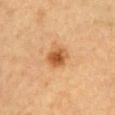Findings:
• follow-up: imaged on a skin check; not biopsied
• patient: female, about 30 years old
• location: the front of the torso
• imaging modality: ~15 mm crop, total-body skin-cancer survey